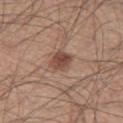The lesion was photographed on a routine skin check and not biopsied; there is no pathology result. The lesion is located on the left thigh. The recorded lesion diameter is about 2.5 mm. A male subject, aged around 45. This image is a 15 mm lesion crop taken from a total-body photograph. An algorithmic analysis of the crop reported about 12 CIELAB-L* units darker than the surrounding skin.